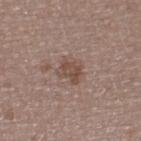<lesion>
  <biopsy_status>not biopsied; imaged during a skin examination</biopsy_status>
  <site>leg</site>
  <patient>
    <sex>female</sex>
    <age_approx>70</age_approx>
  </patient>
  <lighting>white-light</lighting>
  <image>
    <source>total-body photography crop</source>
    <field_of_view_mm>15</field_of_view_mm>
  </image>
</lesion>